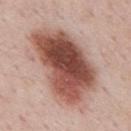notes: no biopsy performed (imaged during a skin exam) | lesion diameter: about 10 mm | subject: male, roughly 50 years of age | tile lighting: white-light | acquisition: ~15 mm crop, total-body skin-cancer survey | anatomic site: the mid back | image-analysis metrics: a lesion area of about 44 mm², an outline eccentricity of about 0.8 (0 = round, 1 = elongated), and two-axis asymmetry of about 0.25.